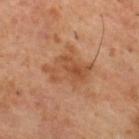Part of a total-body skin-imaging series; this lesion was reviewed on a skin check and was not flagged for biopsy.
The total-body-photography lesion software estimated an area of roughly 13 mm², a shape eccentricity near 0.7, and a symmetry-axis asymmetry near 0.4. The analysis additionally found a lesion color around L≈49 a*≈23 b*≈34 in CIELAB and a normalized lesion–skin contrast near 6.5.
The subject is a male aged around 55.
About 5.5 mm across.
The lesion is on the upper back.
Imaged with cross-polarized lighting.
A lesion tile, about 15 mm wide, cut from a 3D total-body photograph.